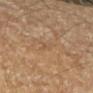automated lesion analysis = an average lesion color of about L≈53 a*≈17 b*≈34 (CIELAB), a lesion–skin lightness drop of about 4, and a normalized border contrast of about 3.5 | image source = ~15 mm tile from a whole-body skin photo | lesion size = ~2.5 mm (longest diameter) | patient = male, roughly 55 years of age | location = the left upper arm | tile lighting = cross-polarized.